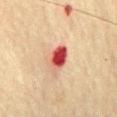Impression: Recorded during total-body skin imaging; not selected for excision or biopsy.